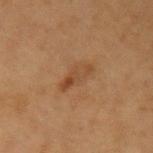Q: Was this lesion biopsied?
A: no biopsy performed (imaged during a skin exam)
Q: What are the patient's age and sex?
A: female, aged around 40
Q: What lighting was used for the tile?
A: cross-polarized
Q: Where on the body is the lesion?
A: the left upper arm
Q: What kind of image is this?
A: total-body-photography crop, ~15 mm field of view
Q: Automated lesion metrics?
A: an eccentricity of roughly 0.9; a border-irregularity rating of about 3/10 and radial color variation of about 1.5; a classifier nevus-likeness of about 10/100 and a detector confidence of about 100 out of 100 that the crop contains a lesion